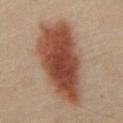Q: Is there a histopathology result?
A: catalogued during a skin exam; not biopsied
Q: Illumination type?
A: cross-polarized illumination
Q: Lesion size?
A: ~11 mm (longest diameter)
Q: What are the patient's age and sex?
A: male, aged approximately 50
Q: What is the imaging modality?
A: 15 mm crop, total-body photography
Q: Lesion location?
A: the chest